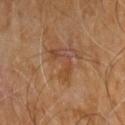No biopsy was performed on this lesion — it was imaged during a full skin examination and was not determined to be concerning. A roughly 15 mm field-of-view crop from a total-body skin photograph. A male patient aged 58 to 62. The lesion is on the arm. The tile uses cross-polarized illumination. Automated image analysis of the tile measured an average lesion color of about L≈43 a*≈20 b*≈31 (CIELAB), roughly 6 lightness units darker than nearby skin, and a normalized lesion–skin contrast near 5.5. And it measured a border-irregularity index near 7/10, a within-lesion color-variation index near 5.5/10, and peripheral color asymmetry of about 2. It also reported a nevus-likeness score of about 0/100.